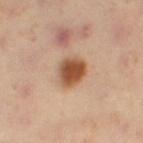Q: Illumination type?
A: cross-polarized
Q: What are the patient's age and sex?
A: female, approximately 40 years of age
Q: What is the anatomic site?
A: the right thigh
Q: Lesion size?
A: ≈3.5 mm
Q: What kind of image is this?
A: total-body-photography crop, ~15 mm field of view